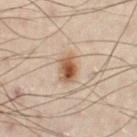workup: imaged on a skin check; not biopsied
tile lighting: cross-polarized
diameter: ~3.5 mm (longest diameter)
patient: male, approximately 50 years of age
location: the left lower leg
image: total-body-photography crop, ~15 mm field of view